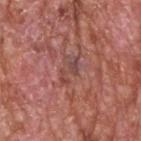Part of a total-body skin-imaging series; this lesion was reviewed on a skin check and was not flagged for biopsy.
On the head or neck.
This image is a 15 mm lesion crop taken from a total-body photograph.
The patient is a male aged approximately 60.
Approximately 3.5 mm at its widest.